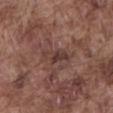No biopsy was performed on this lesion — it was imaged during a full skin examination and was not determined to be concerning.
The lesion is located on the abdomen.
A 15 mm close-up tile from a total-body photography series done for melanoma screening.
Imaged with white-light lighting.
Automated image analysis of the tile measured a mean CIELAB color near L≈37 a*≈19 b*≈21, about 8 CIELAB-L* units darker than the surrounding skin, and a lesion-to-skin contrast of about 7 (normalized; higher = more distinct). The software also gave border irregularity of about 3.5 on a 0–10 scale and a within-lesion color-variation index near 2.5/10. It also reported an automated nevus-likeness rating near 5 out of 100 and lesion-presence confidence of about 85/100.
A male subject approximately 75 years of age.